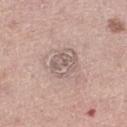Clinical summary:
This image is a 15 mm lesion crop taken from a total-body photograph. On the right lower leg. The tile uses white-light illumination. An algorithmic analysis of the crop reported a footprint of about 7.5 mm², an outline eccentricity of about 0.55 (0 = round, 1 = elongated), and a symmetry-axis asymmetry near 0.25. The software also gave about 8 CIELAB-L* units darker than the surrounding skin. The analysis additionally found peripheral color asymmetry of about 1.5. It also reported a classifier nevus-likeness of about 0/100 and a lesion-detection confidence of about 90/100. A male patient, roughly 65 years of age. Measured at roughly 3.5 mm in maximum diameter.